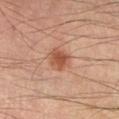Clinical summary: The lesion is on the right forearm. This image is a 15 mm lesion crop taken from a total-body photograph. The patient is a male roughly 40 years of age.